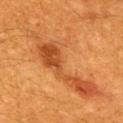This lesion was catalogued during total-body skin photography and was not selected for biopsy. On the upper back. The tile uses cross-polarized illumination. Cropped from a total-body skin-imaging series; the visible field is about 15 mm. Approximately 14 mm at its widest. The total-body-photography lesion software estimated an average lesion color of about L≈50 a*≈27 b*≈42 (CIELAB), a lesion–skin lightness drop of about 8, and a lesion-to-skin contrast of about 6 (normalized; higher = more distinct). A male patient aged approximately 60.